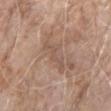Assessment: Imaged during a routine full-body skin examination; the lesion was not biopsied and no histopathology is available. Context: The total-body-photography lesion software estimated a lesion color around L≈54 a*≈17 b*≈27 in CIELAB, a lesion–skin lightness drop of about 6, and a lesion-to-skin contrast of about 4.5 (normalized; higher = more distinct). And it measured a lesion-detection confidence of about 80/100. Imaged with white-light lighting. About 4 mm across. A roughly 15 mm field-of-view crop from a total-body skin photograph. Located on the right upper arm. The subject is a female aged approximately 85 or older.